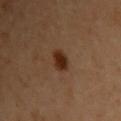Assessment:
Part of a total-body skin-imaging series; this lesion was reviewed on a skin check and was not flagged for biopsy.
Background:
A close-up tile cropped from a whole-body skin photograph, about 15 mm across. The lesion is on the upper back. Imaged with cross-polarized lighting. Measured at roughly 3 mm in maximum diameter. The subject is a female aged 58 to 62.